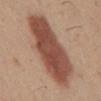  biopsy_status: not biopsied; imaged during a skin examination
  site: abdomen
  lighting: white-light
  image:
    source: total-body photography crop
    field_of_view_mm: 15
  patient:
    sex: male
    age_approx: 30
  automated_metrics:
    area_mm2_approx: 40.0
    shape_asymmetry: 0.15
    cielab_L: 49
    cielab_a: 22
    cielab_b: 28
    vs_skin_darker_L: 15.0
    vs_skin_contrast_norm: 10.5
    border_irregularity_0_10: 2.5
    color_variation_0_10: 4.5
    peripheral_color_asymmetry: 1.5
    nevus_likeness_0_100: 100
    lesion_detection_confidence_0_100: 100
  lesion_size:
    long_diameter_mm_approx: 10.5